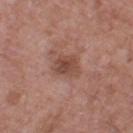Recorded during total-body skin imaging; not selected for excision or biopsy.